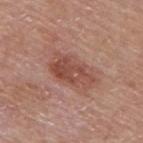A lesion tile, about 15 mm wide, cut from a 3D total-body photograph. The lesion is on the upper back. Automated image analysis of the tile measured about 9 CIELAB-L* units darker than the surrounding skin and a normalized lesion–skin contrast near 7. A male subject, roughly 80 years of age. Measured at roughly 6 mm in maximum diameter. Captured under white-light illumination.